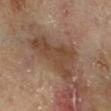Assessment:
The lesion was photographed on a routine skin check and not biopsied; there is no pathology result.
Context:
A close-up tile cropped from a whole-body skin photograph, about 15 mm across. From the right lower leg. A male patient, in their mid-60s.